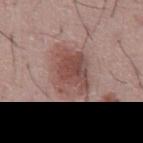Part of a total-body skin-imaging series; this lesion was reviewed on a skin check and was not flagged for biopsy.
About 5.5 mm across.
The tile uses white-light illumination.
From the abdomen.
The total-body-photography lesion software estimated a lesion color around L≈49 a*≈21 b*≈22 in CIELAB, a lesion–skin lightness drop of about 11, and a normalized border contrast of about 8. And it measured an automated nevus-likeness rating near 95 out of 100 and a lesion-detection confidence of about 100/100.
A male patient, aged approximately 45.
Cropped from a whole-body photographic skin survey; the tile spans about 15 mm.